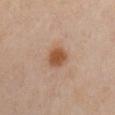Part of a total-body skin-imaging series; this lesion was reviewed on a skin check and was not flagged for biopsy. The lesion is located on the chest. Cropped from a total-body skin-imaging series; the visible field is about 15 mm. Approximately 3 mm at its widest. A female subject about 40 years old.